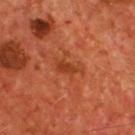follow-up: total-body-photography surveillance lesion; no biopsy | tile lighting: cross-polarized | size: ≈3.5 mm | acquisition: ~15 mm crop, total-body skin-cancer survey | TBP lesion metrics: a lesion color around L≈38 a*≈30 b*≈37 in CIELAB, a lesion–skin lightness drop of about 7, and a lesion-to-skin contrast of about 6.5 (normalized; higher = more distinct); border irregularity of about 4.5 on a 0–10 scale, a within-lesion color-variation index near 1.5/10, and radial color variation of about 0.5; a classifier nevus-likeness of about 0/100 and lesion-presence confidence of about 100/100 | subject: male, aged 53–57 | location: the chest.